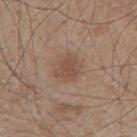Impression:
The lesion was tiled from a total-body skin photograph and was not biopsied.
Background:
The lesion is on the mid back. Imaged with white-light lighting. An algorithmic analysis of the crop reported an average lesion color of about L≈49 a*≈18 b*≈27 (CIELAB), about 8 CIELAB-L* units darker than the surrounding skin, and a normalized border contrast of about 6. It also reported a border-irregularity index near 2.5/10 and a color-variation rating of about 2/10. The patient is a male aged around 45. The lesion's longest dimension is about 3 mm. A 15 mm close-up extracted from a 3D total-body photography capture.